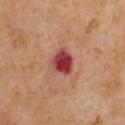Clinical impression: Recorded during total-body skin imaging; not selected for excision or biopsy. Background: Longest diameter approximately 3.5 mm. A 15 mm crop from a total-body photograph taken for skin-cancer surveillance. The subject is a female aged around 70. Located on the upper back. The tile uses cross-polarized illumination.